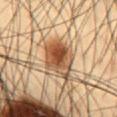notes — catalogued during a skin exam; not biopsied | size — ~4.5 mm (longest diameter) | image-analysis metrics — internal color variation of about 8 on a 0–10 scale and peripheral color asymmetry of about 2.5 | acquisition — ~15 mm tile from a whole-body skin photo | subject — male, roughly 55 years of age | illumination — cross-polarized illumination | body site — the front of the torso.